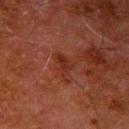site: the right upper arm | tile lighting: cross-polarized | size: ~2.5 mm (longest diameter) | patient: male, roughly 80 years of age | image: ~15 mm crop, total-body skin-cancer survey.